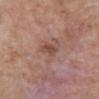Notes:
* subject · male, aged 58–62
* body site · the front of the torso
* imaging modality · ~15 mm crop, total-body skin-cancer survey
* TBP lesion metrics · border irregularity of about 2.5 on a 0–10 scale, a within-lesion color-variation index near 1.5/10, and radial color variation of about 0.5